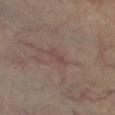Recorded during total-body skin imaging; not selected for excision or biopsy. Located on the left lower leg. A region of skin cropped from a whole-body photographic capture, roughly 15 mm wide. A male subject, aged 58 to 62. Longest diameter approximately 3 mm.